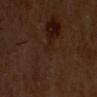| feature | finding |
|---|---|
| follow-up | total-body-photography surveillance lesion; no biopsy |
| subject | male, aged around 60 |
| size | ~1.5 mm (longest diameter) |
| body site | the head or neck |
| illumination | cross-polarized |
| image | ~15 mm crop, total-body skin-cancer survey |